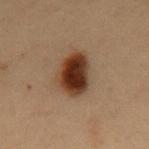Findings:
– biopsy status — catalogued during a skin exam; not biopsied
– imaging modality — 15 mm crop, total-body photography
– subject — male, about 55 years old
– lesion diameter — about 5 mm
– automated metrics — roughly 15 lightness units darker than nearby skin and a normalized border contrast of about 14.5; a border-irregularity index near 2/10, internal color variation of about 5.5 on a 0–10 scale, and radial color variation of about 1.5; a classifier nevus-likeness of about 100/100
– location — the mid back
– tile lighting — cross-polarized